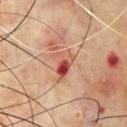Clinical impression:
No biopsy was performed on this lesion — it was imaged during a full skin examination and was not determined to be concerning.
Image and clinical context:
A male subject, about 70 years old. A 15 mm close-up tile from a total-body photography series done for melanoma screening. The lesion's longest dimension is about 3.5 mm. The total-body-photography lesion software estimated a mean CIELAB color near L≈52 a*≈28 b*≈31, a lesion–skin lightness drop of about 12, and a lesion-to-skin contrast of about 8.5 (normalized; higher = more distinct). This is a cross-polarized tile. The lesion is on the chest.